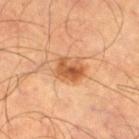Recorded during total-body skin imaging; not selected for excision or biopsy. A male patient aged around 65. A region of skin cropped from a whole-body photographic capture, roughly 15 mm wide. The lesion is located on the left thigh.